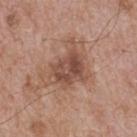No biopsy was performed on this lesion — it was imaged during a full skin examination and was not determined to be concerning.
This image is a 15 mm lesion crop taken from a total-body photograph.
Automated tile analysis of the lesion measured a lesion area of about 17 mm², an eccentricity of roughly 0.65, and a shape-asymmetry score of about 0.45 (0 = symmetric). The analysis additionally found a mean CIELAB color near L≈50 a*≈21 b*≈27, a lesion–skin lightness drop of about 10, and a lesion-to-skin contrast of about 7.5 (normalized; higher = more distinct). It also reported a classifier nevus-likeness of about 15/100 and a lesion-detection confidence of about 100/100.
Approximately 6 mm at its widest.
The tile uses white-light illumination.
The lesion is on the upper back.
A male subject in their mid-60s.